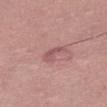Clinical impression: The lesion was photographed on a routine skin check and not biopsied; there is no pathology result. Context: Automated image analysis of the tile measured an area of roughly 3.5 mm². The software also gave border irregularity of about 5 on a 0–10 scale, a color-variation rating of about 0.5/10, and peripheral color asymmetry of about 0. Cropped from a whole-body photographic skin survey; the tile spans about 15 mm. Captured under white-light illumination. About 3 mm across. A male patient, aged around 60. From the right thigh.